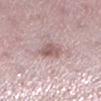biopsy status = imaged on a skin check; not biopsied
subject = female, in their mid- to late 40s
location = the left lower leg
acquisition = 15 mm crop, total-body photography